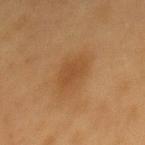Captured during whole-body skin photography for melanoma surveillance; the lesion was not biopsied.